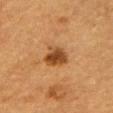Part of a total-body skin-imaging series; this lesion was reviewed on a skin check and was not flagged for biopsy. The lesion's longest dimension is about 3 mm. From the arm. The subject is a male approximately 85 years of age or older. A 15 mm close-up tile from a total-body photography series done for melanoma screening. Captured under cross-polarized illumination. The total-body-photography lesion software estimated a mean CIELAB color near L≈40 a*≈21 b*≈36, roughly 12 lightness units darker than nearby skin, and a lesion-to-skin contrast of about 10 (normalized; higher = more distinct).